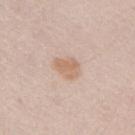A female patient, approximately 50 years of age. The recorded lesion diameter is about 3.5 mm. Automated image analysis of the tile measured a footprint of about 6 mm², a shape eccentricity near 0.75, and a shape-asymmetry score of about 0.25 (0 = symmetric). The software also gave a mean CIELAB color near L≈65 a*≈18 b*≈30, a lesion–skin lightness drop of about 8, and a normalized border contrast of about 6.5. A roughly 15 mm field-of-view crop from a total-body skin photograph. The lesion is located on the right upper arm. Imaged with white-light lighting.